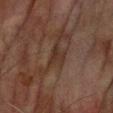Impression:
Recorded during total-body skin imaging; not selected for excision or biopsy.
Acquisition and patient details:
The tile uses cross-polarized illumination. A region of skin cropped from a whole-body photographic capture, roughly 15 mm wide. Located on the right forearm. Automated image analysis of the tile measured a lesion color around L≈25 a*≈13 b*≈20 in CIELAB, roughly 5 lightness units darker than nearby skin, and a normalized border contrast of about 6. The analysis additionally found an automated nevus-likeness rating near 0 out of 100 and a lesion-detection confidence of about 65/100. The subject is a male in their mid- to late 70s. Longest diameter approximately 3 mm.